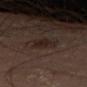notes = imaged on a skin check; not biopsied | diameter = ~2.5 mm (longest diameter) | body site = the left thigh | imaging modality = ~15 mm tile from a whole-body skin photo | lighting = cross-polarized illumination.